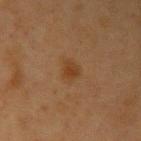Part of a total-body skin-imaging series; this lesion was reviewed on a skin check and was not flagged for biopsy. Measured at roughly 2.5 mm in maximum diameter. A female patient, in their 40s. The total-body-photography lesion software estimated a lesion-to-skin contrast of about 6 (normalized; higher = more distinct). The software also gave border irregularity of about 2.5 on a 0–10 scale, internal color variation of about 2 on a 0–10 scale, and a peripheral color-asymmetry measure near 0.5. A 15 mm crop from a total-body photograph taken for skin-cancer surveillance. The tile uses cross-polarized illumination. On the arm.